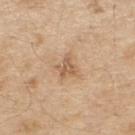Part of a total-body skin-imaging series; this lesion was reviewed on a skin check and was not flagged for biopsy. A male patient roughly 70 years of age. A region of skin cropped from a whole-body photographic capture, roughly 15 mm wide. From the back. The recorded lesion diameter is about 2.5 mm. The tile uses white-light illumination.